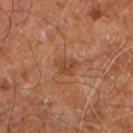No biopsy was performed on this lesion — it was imaged during a full skin examination and was not determined to be concerning.
The recorded lesion diameter is about 3 mm.
A male subject in their 60s.
On the right leg.
A 15 mm crop from a total-body photograph taken for skin-cancer surveillance.
Automated tile analysis of the lesion measured a lesion area of about 4 mm² and an eccentricity of roughly 0.7. The software also gave lesion-presence confidence of about 100/100.